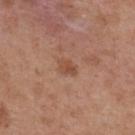Captured during whole-body skin photography for melanoma surveillance; the lesion was not biopsied. A 15 mm close-up tile from a total-body photography series done for melanoma screening. The lesion is located on the upper back. A female subject approximately 40 years of age.